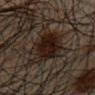Clinical impression:
Imaged during a routine full-body skin examination; the lesion was not biopsied and no histopathology is available.
Acquisition and patient details:
This image is a 15 mm lesion crop taken from a total-body photograph. Approximately 5 mm at its widest. The patient is a male aged around 65. Imaged with cross-polarized lighting. An algorithmic analysis of the crop reported an area of roughly 16 mm², an outline eccentricity of about 0.65 (0 = round, 1 = elongated), and two-axis asymmetry of about 0.35. The analysis additionally found about 9 CIELAB-L* units darker than the surrounding skin. From the front of the torso.